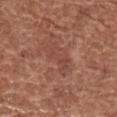biopsy status = no biopsy performed (imaged during a skin exam); anatomic site = the left forearm; subject = female, aged 48–52; image = ~15 mm crop, total-body skin-cancer survey.